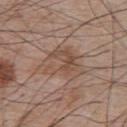Part of a total-body skin-imaging series; this lesion was reviewed on a skin check and was not flagged for biopsy. The lesion is on the back. A 15 mm close-up extracted from a 3D total-body photography capture. Longest diameter approximately 6.5 mm. The patient is a male in their mid- to late 60s. This is a white-light tile.